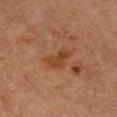Imaged during a routine full-body skin examination; the lesion was not biopsied and no histopathology is available.
A female patient in their mid- to late 40s.
Automated image analysis of the tile measured a mean CIELAB color near L≈38 a*≈20 b*≈32, a lesion–skin lightness drop of about 6, and a lesion-to-skin contrast of about 6.5 (normalized; higher = more distinct). The software also gave a border-irregularity rating of about 3.5/10, a within-lesion color-variation index near 3/10, and radial color variation of about 1.
A close-up tile cropped from a whole-body skin photograph, about 15 mm across.
On the chest.
Longest diameter approximately 4.5 mm.
Imaged with cross-polarized lighting.